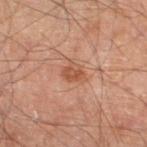biopsy status = total-body-photography surveillance lesion; no biopsy | automated metrics = a nevus-likeness score of about 5/100 and lesion-presence confidence of about 100/100 | tile lighting = cross-polarized | size = ~2.5 mm (longest diameter) | patient = male, in their mid- to late 60s | imaging modality = ~15 mm crop, total-body skin-cancer survey | location = the left thigh.